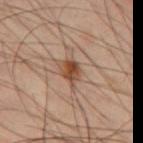biopsy status = catalogued during a skin exam; not biopsied | subject = male, aged around 50 | image = ~15 mm crop, total-body skin-cancer survey | lesion size = ~3 mm (longest diameter) | automated lesion analysis = border irregularity of about 3.5 on a 0–10 scale, internal color variation of about 5.5 on a 0–10 scale, and peripheral color asymmetry of about 2 | lighting = cross-polarized illumination | body site = the leg.